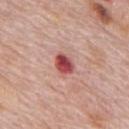Assessment:
The lesion was tiled from a total-body skin photograph and was not biopsied.
Clinical summary:
Automated tile analysis of the lesion measured a footprint of about 5 mm² and a symmetry-axis asymmetry near 0.25. And it measured roughly 17 lightness units darker than nearby skin and a lesion-to-skin contrast of about 11.5 (normalized; higher = more distinct). It also reported a within-lesion color-variation index near 5/10 and radial color variation of about 2. The lesion's longest dimension is about 3 mm. A male subject, aged around 75. Cropped from a whole-body photographic skin survey; the tile spans about 15 mm. Located on the back.